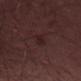Clinical impression: Recorded during total-body skin imaging; not selected for excision or biopsy. Image and clinical context: A male subject aged 48–52. Located on the lower back. A region of skin cropped from a whole-body photographic capture, roughly 15 mm wide.